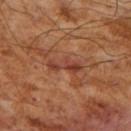• workup · imaged on a skin check; not biopsied